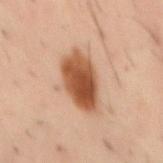Q: Was a biopsy performed?
A: catalogued during a skin exam; not biopsied
Q: What kind of image is this?
A: ~15 mm crop, total-body skin-cancer survey
Q: Illumination type?
A: cross-polarized illumination
Q: Lesion location?
A: the mid back
Q: What did automated image analysis measure?
A: a mean CIELAB color near L≈52 a*≈24 b*≈34 and a lesion–skin lightness drop of about 17; a border-irregularity index near 2.5/10, a within-lesion color-variation index near 6.5/10, and peripheral color asymmetry of about 2; an automated nevus-likeness rating near 100 out of 100 and a lesion-detection confidence of about 100/100
Q: What are the patient's age and sex?
A: male, roughly 40 years of age
Q: How large is the lesion?
A: about 6.5 mm